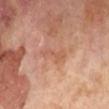Impression: No biopsy was performed on this lesion — it was imaged during a full skin examination and was not determined to be concerning. Clinical summary: This is a cross-polarized tile. A male patient, in their 70s. Located on the left lower leg. A lesion tile, about 15 mm wide, cut from a 3D total-body photograph.